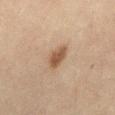{"lighting": "cross-polarized", "site": "mid back", "automated_metrics": {"area_mm2_approx": 5.5, "shape_asymmetry": 0.2, "border_irregularity_0_10": 2.0, "color_variation_0_10": 2.5, "peripheral_color_asymmetry": 1.0, "nevus_likeness_0_100": 90, "lesion_detection_confidence_0_100": 100}, "patient": {"sex": "female", "age_approx": 60}, "image": {"source": "total-body photography crop", "field_of_view_mm": 15}}